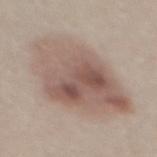notes: imaged on a skin check; not biopsied | patient: male, aged 28 to 32 | automated metrics: a lesion area of about 49 mm², an outline eccentricity of about 0.7 (0 = round, 1 = elongated), and two-axis asymmetry of about 0.15 | lesion diameter: about 9.5 mm | image: 15 mm crop, total-body photography | location: the mid back | illumination: white-light illumination.